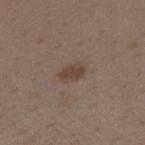The subject is a female aged 33 to 37.
Located on the mid back.
A lesion tile, about 15 mm wide, cut from a 3D total-body photograph.
Approximately 3 mm at its widest.
Automated image analysis of the tile measured an area of roughly 4.5 mm² and a shape eccentricity near 0.8. The analysis additionally found an average lesion color of about L≈42 a*≈15 b*≈24 (CIELAB) and roughly 8 lightness units darker than nearby skin. It also reported internal color variation of about 2 on a 0–10 scale.